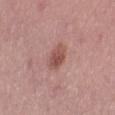biopsy status = no biopsy performed (imaged during a skin exam)
TBP lesion metrics = a detector confidence of about 100 out of 100 that the crop contains a lesion
anatomic site = the right thigh
diameter = ~3.5 mm (longest diameter)
subject = male, in their 40s
image source = ~15 mm tile from a whole-body skin photo
lighting = white-light illumination